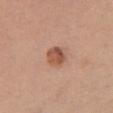follow-up: catalogued during a skin exam; not biopsied | body site: the chest | subject: female, about 45 years old | TBP lesion metrics: a lesion area of about 5.5 mm², an outline eccentricity of about 0.5 (0 = round, 1 = elongated), and a symmetry-axis asymmetry near 0.15; a mean CIELAB color near L≈53 a*≈23 b*≈30; a border-irregularity rating of about 1.5/10, a within-lesion color-variation index near 5/10, and radial color variation of about 1.5 | lighting: white-light illumination | image: 15 mm crop, total-body photography.